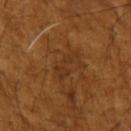notes: no biopsy performed (imaged during a skin exam) | acquisition: 15 mm crop, total-body photography | tile lighting: cross-polarized | subject: male, aged 63 to 67 | automated metrics: a lesion color around L≈33 a*≈21 b*≈34 in CIELAB, roughly 6 lightness units darker than nearby skin, and a lesion-to-skin contrast of about 5.5 (normalized; higher = more distinct); a border-irregularity index near 7.5/10, internal color variation of about 0.5 on a 0–10 scale, and peripheral color asymmetry of about 0; an automated nevus-likeness rating near 0 out of 100 | location: the left upper arm | diameter: ≈4.5 mm.